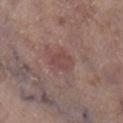Longest diameter approximately 3 mm. From the left lower leg. The total-body-photography lesion software estimated an average lesion color of about L≈45 a*≈20 b*≈20 (CIELAB), roughly 7 lightness units darker than nearby skin, and a lesion-to-skin contrast of about 5.5 (normalized; higher = more distinct). It also reported border irregularity of about 2 on a 0–10 scale, a within-lesion color-variation index near 1.5/10, and peripheral color asymmetry of about 0.5. A lesion tile, about 15 mm wide, cut from a 3D total-body photograph. Captured under white-light illumination. A female patient, aged around 85 or older.